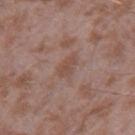Recorded during total-body skin imaging; not selected for excision or biopsy. The total-body-photography lesion software estimated a lesion area of about 3.5 mm², an outline eccentricity of about 0.85 (0 = round, 1 = elongated), and a shape-asymmetry score of about 0.3 (0 = symmetric). The analysis additionally found an average lesion color of about L≈48 a*≈18 b*≈25 (CIELAB). The analysis additionally found a border-irregularity rating of about 3/10, a color-variation rating of about 1.5/10, and radial color variation of about 0.5. A 15 mm close-up extracted from a 3D total-body photography capture. The lesion's longest dimension is about 3 mm. A male subject aged approximately 45. Imaged with white-light lighting. The lesion is located on the leg.